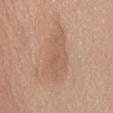{"biopsy_status": "not biopsied; imaged during a skin examination"}